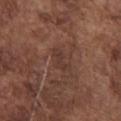{"biopsy_status": "not biopsied; imaged during a skin examination", "lesion_size": {"long_diameter_mm_approx": 3.0}, "patient": {"sex": "male", "age_approx": 75}, "lighting": "white-light", "site": "chest", "image": {"source": "total-body photography crop", "field_of_view_mm": 15}}